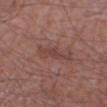Q: Where on the body is the lesion?
A: the arm
Q: What is the imaging modality?
A: ~15 mm tile from a whole-body skin photo
Q: What are the patient's age and sex?
A: male, in their 50s